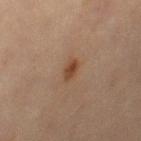The lesion was tiled from a total-body skin photograph and was not biopsied. Captured under cross-polarized illumination. The patient is a female roughly 70 years of age. Automated image analysis of the tile measured a classifier nevus-likeness of about 95/100 and lesion-presence confidence of about 100/100. The lesion is on the right thigh. Cropped from a whole-body photographic skin survey; the tile spans about 15 mm. About 2.5 mm across.